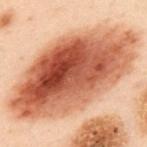workup = catalogued during a skin exam; not biopsied.